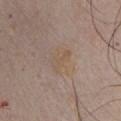Q: What lighting was used for the tile?
A: white-light illumination
Q: What is the imaging modality?
A: 15 mm crop, total-body photography
Q: Lesion location?
A: the chest
Q: Patient demographics?
A: male, aged 43–47
Q: What did automated image analysis measure?
A: a mean CIELAB color near L≈53 a*≈14 b*≈27 and roughly 4 lightness units darker than nearby skin; a border-irregularity rating of about 5.5/10 and a color-variation rating of about 0/10
Q: How large is the lesion?
A: ≈3 mm